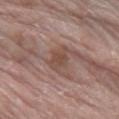Clinical impression:
Part of a total-body skin-imaging series; this lesion was reviewed on a skin check and was not flagged for biopsy.
Image and clinical context:
The lesion-visualizer software estimated a lesion color around L≈47 a*≈18 b*≈24 in CIELAB. The analysis additionally found lesion-presence confidence of about 100/100. Measured at roughly 3.5 mm in maximum diameter. From the right lower leg. This is a white-light tile. A female subject, aged 68 to 72. A region of skin cropped from a whole-body photographic capture, roughly 15 mm wide.